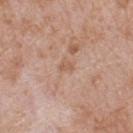notes = no biopsy performed (imaged during a skin exam); location = the upper back; subject = male, roughly 65 years of age; illumination = white-light illumination; lesion size = about 2 mm; image = ~15 mm crop, total-body skin-cancer survey.